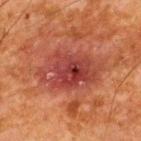Captured during whole-body skin photography for melanoma surveillance; the lesion was not biopsied. On the upper back. Longest diameter approximately 7 mm. The subject is a male aged 63–67. A 15 mm crop from a total-body photograph taken for skin-cancer surveillance.